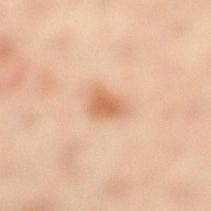| feature | finding |
|---|---|
| follow-up | no biopsy performed (imaged during a skin exam) |
| location | the right leg |
| subject | female, aged approximately 40 |
| imaging modality | 15 mm crop, total-body photography |